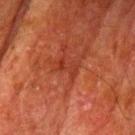No biopsy was performed on this lesion — it was imaged during a full skin examination and was not determined to be concerning.
The tile uses cross-polarized illumination.
Cropped from a total-body skin-imaging series; the visible field is about 15 mm.
Located on the left upper arm.
Approximately 2.5 mm at its widest.
A male subject, aged around 80.
An algorithmic analysis of the crop reported an average lesion color of about L≈30 a*≈28 b*≈29 (CIELAB), about 5 CIELAB-L* units darker than the surrounding skin, and a normalized lesion–skin contrast near 5.